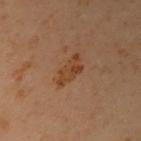Context:
Cropped from a total-body skin-imaging series; the visible field is about 15 mm. On the chest. Approximately 4 mm at its widest. A female subject, approximately 60 years of age.